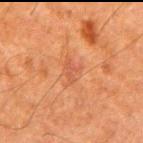Part of a total-body skin-imaging series; this lesion was reviewed on a skin check and was not flagged for biopsy.
A 15 mm close-up extracted from a 3D total-body photography capture.
The patient is a male aged approximately 60.
Longest diameter approximately 2.5 mm.
Imaged with cross-polarized lighting.
The lesion is located on the right upper arm.
Automated image analysis of the tile measured roughly 6 lightness units darker than nearby skin and a normalized lesion–skin contrast near 5.